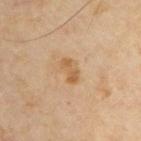The lesion was photographed on a routine skin check and not biopsied; there is no pathology result. Captured under cross-polarized illumination. An algorithmic analysis of the crop reported a mean CIELAB color near L≈54 a*≈17 b*≈36, a lesion–skin lightness drop of about 8, and a normalized lesion–skin contrast near 7. The analysis additionally found a nevus-likeness score of about 5/100. A male subject, about 55 years old. A close-up tile cropped from a whole-body skin photograph, about 15 mm across. The lesion is on the left upper arm. About 3 mm across.